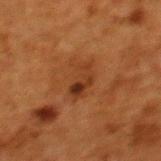No biopsy was performed on this lesion — it was imaged during a full skin examination and was not determined to be concerning. A 15 mm close-up extracted from a 3D total-body photography capture. A female patient, in their 50s. The lesion is located on the upper back.